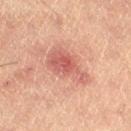Recorded during total-body skin imaging; not selected for excision or biopsy. Approximately 5 mm at its widest. The tile uses cross-polarized illumination. This image is a 15 mm lesion crop taken from a total-body photograph. Automated tile analysis of the lesion measured a footprint of about 12 mm², an outline eccentricity of about 0.8 (0 = round, 1 = elongated), and a shape-asymmetry score of about 0.4 (0 = symmetric). The subject is a male aged around 60.